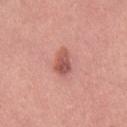No biopsy was performed on this lesion — it was imaged during a full skin examination and was not determined to be concerning.
The tile uses white-light illumination.
Automated image analysis of the tile measured a mean CIELAB color near L≈55 a*≈27 b*≈27, a lesion–skin lightness drop of about 12, and a normalized border contrast of about 8. It also reported a color-variation rating of about 4.5/10 and peripheral color asymmetry of about 1.5. And it measured a lesion-detection confidence of about 100/100.
Measured at roughly 3.5 mm in maximum diameter.
The lesion is on the right thigh.
A 15 mm close-up tile from a total-body photography series done for melanoma screening.
The subject is a female aged approximately 30.